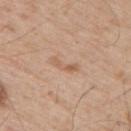Clinical impression: No biopsy was performed on this lesion — it was imaged during a full skin examination and was not determined to be concerning. Clinical summary: A male patient in their mid-50s. A close-up tile cropped from a whole-body skin photograph, about 15 mm across. On the upper back. Approximately 3.5 mm at its widest.